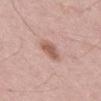{
  "image": {
    "source": "total-body photography crop",
    "field_of_view_mm": 15
  },
  "site": "abdomen",
  "lighting": "white-light",
  "automated_metrics": {
    "area_mm2_approx": 6.0,
    "shape_asymmetry": 0.2,
    "cielab_L": 58,
    "cielab_a": 21,
    "cielab_b": 26,
    "vs_skin_contrast_norm": 7.5,
    "border_irregularity_0_10": 2.0,
    "peripheral_color_asymmetry": 0.5
  },
  "lesion_size": {
    "long_diameter_mm_approx": 3.0
  },
  "patient": {
    "sex": "male",
    "age_approx": 55
  }
}